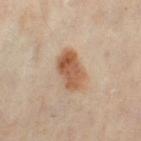{"lighting": "cross-polarized", "patient": {"sex": "female", "age_approx": 60}, "image": {"source": "total-body photography crop", "field_of_view_mm": 15}, "lesion_size": {"long_diameter_mm_approx": 4.5}, "site": "leg"}